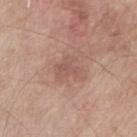Assessment: No biopsy was performed on this lesion — it was imaged during a full skin examination and was not determined to be concerning. Clinical summary: The recorded lesion diameter is about 3.5 mm. A male subject, aged 58 to 62. Cropped from a whole-body photographic skin survey; the tile spans about 15 mm. On the right upper arm. The tile uses white-light illumination.